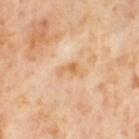{"biopsy_status": "not biopsied; imaged during a skin examination", "image": {"source": "total-body photography crop", "field_of_view_mm": 15}, "site": "right thigh", "automated_metrics": {"cielab_L": 68, "cielab_a": 21, "cielab_b": 40, "vs_skin_darker_L": 8.0, "vs_skin_contrast_norm": 6.0, "border_irregularity_0_10": 4.5, "color_variation_0_10": 1.0, "peripheral_color_asymmetry": 0.0}, "patient": {"sex": "female", "age_approx": 55}}